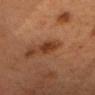  biopsy_status: not biopsied; imaged during a skin examination
  patient:
    sex: female
    age_approx: 40
  image:
    source: total-body photography crop
    field_of_view_mm: 15
  automated_metrics:
    vs_skin_darker_L: 9.0
    vs_skin_contrast_norm: 9.0
  site: head or neck